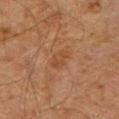{"biopsy_status": "not biopsied; imaged during a skin examination", "automated_metrics": {"cielab_L": 35, "cielab_a": 18, "cielab_b": 28, "vs_skin_darker_L": 4.0, "nevus_likeness_0_100": 0}, "site": "chest", "image": {"source": "total-body photography crop", "field_of_view_mm": 15}, "patient": {"sex": "male", "age_approx": 60}}